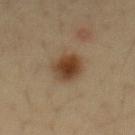No biopsy was performed on this lesion — it was imaged during a full skin examination and was not determined to be concerning. On the abdomen. A male subject, aged around 35. A 15 mm crop from a total-body photograph taken for skin-cancer surveillance. An algorithmic analysis of the crop reported a nevus-likeness score of about 100/100 and a detector confidence of about 100 out of 100 that the crop contains a lesion.